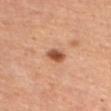- workup: no biopsy performed (imaged during a skin exam)
- patient: female
- site: the abdomen
- image: ~15 mm tile from a whole-body skin photo